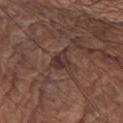<tbp_lesion>
<biopsy_status>not biopsied; imaged during a skin examination</biopsy_status>
<site>front of the torso</site>
<patient>
  <sex>male</sex>
  <age_approx>75</age_approx>
</patient>
<image>
  <source>total-body photography crop</source>
  <field_of_view_mm>15</field_of_view_mm>
</image>
<automated_metrics>
  <cielab_L>33</cielab_L>
  <cielab_a>17</cielab_a>
  <cielab_b>20</cielab_b>
  <vs_skin_darker_L>8.0</vs_skin_darker_L>
  <vs_skin_contrast_norm>8.0</vs_skin_contrast_norm>
  <border_irregularity_0_10>3.5</border_irregularity_0_10>
  <color_variation_0_10>4.0</color_variation_0_10>
  <peripheral_color_asymmetry>1.5</peripheral_color_asymmetry>
  <nevus_likeness_0_100>0</nevus_likeness_0_100>
  <lesion_detection_confidence_0_100>55</lesion_detection_confidence_0_100>
</automated_metrics>
<lesion_size>
  <long_diameter_mm_approx>3.0</long_diameter_mm_approx>
</lesion_size>
</tbp_lesion>